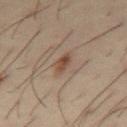<lesion>
<biopsy_status>not biopsied; imaged during a skin examination</biopsy_status>
<lesion_size>
  <long_diameter_mm_approx>3.0</long_diameter_mm_approx>
</lesion_size>
<lighting>cross-polarized</lighting>
<patient>
  <sex>male</sex>
  <age_approx>40</age_approx>
</patient>
<site>chest</site>
<image>
  <source>total-body photography crop</source>
  <field_of_view_mm>15</field_of_view_mm>
</image>
</lesion>